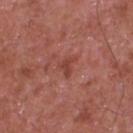Acquisition and patient details: The lesion's longest dimension is about 2.5 mm. The patient is a male aged 63–67. The total-body-photography lesion software estimated roughly 7 lightness units darker than nearby skin and a lesion-to-skin contrast of about 6 (normalized; higher = more distinct). It also reported border irregularity of about 5.5 on a 0–10 scale, a within-lesion color-variation index near 1/10, and a peripheral color-asymmetry measure near 0.5. The lesion is on the chest. A lesion tile, about 15 mm wide, cut from a 3D total-body photograph.